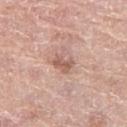Impression: Captured during whole-body skin photography for melanoma surveillance; the lesion was not biopsied. Acquisition and patient details: A female subject, aged approximately 60. A 15 mm close-up tile from a total-body photography series done for melanoma screening. Automated image analysis of the tile measured a lesion area of about 4 mm², an eccentricity of roughly 0.7, and a shape-asymmetry score of about 0.25 (0 = symmetric). It also reported a border-irregularity rating of about 3/10, internal color variation of about 2 on a 0–10 scale, and peripheral color asymmetry of about 0.5. The software also gave an automated nevus-likeness rating near 10 out of 100 and a detector confidence of about 100 out of 100 that the crop contains a lesion. The lesion is located on the right thigh.